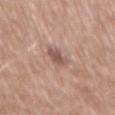The lesion was tiled from a total-body skin photograph and was not biopsied. The tile uses white-light illumination. Measured at roughly 3 mm in maximum diameter. The lesion-visualizer software estimated border irregularity of about 3 on a 0–10 scale, a color-variation rating of about 3.5/10, and a peripheral color-asymmetry measure near 1. The lesion is located on the back. A male subject, approximately 70 years of age. Cropped from a total-body skin-imaging series; the visible field is about 15 mm.